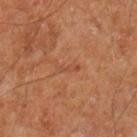workup: no biopsy performed (imaged during a skin exam)
tile lighting: cross-polarized illumination
diameter: ≈3 mm
TBP lesion metrics: a mean CIELAB color near L≈46 a*≈25 b*≈33 and roughly 6 lightness units darker than nearby skin; radial color variation of about 0
patient: male, in their mid- to late 60s
anatomic site: the left upper arm
image source: 15 mm crop, total-body photography